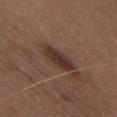Image and clinical context: A female subject aged 48 to 52. A 15 mm close-up tile from a total-body photography series done for melanoma screening. The lesion is located on the chest.